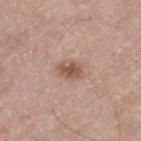Background: This image is a 15 mm lesion crop taken from a total-body photograph. A male subject approximately 65 years of age. From the left thigh.